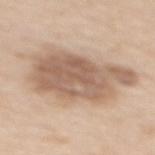Q: Automated lesion metrics?
A: an average lesion color of about L≈60 a*≈16 b*≈29 (CIELAB), about 13 CIELAB-L* units darker than the surrounding skin, and a normalized lesion–skin contrast near 8; a classifier nevus-likeness of about 10/100 and a lesion-detection confidence of about 100/100
Q: Illumination type?
A: white-light
Q: What kind of image is this?
A: total-body-photography crop, ~15 mm field of view
Q: Lesion location?
A: the mid back
Q: Who is the patient?
A: female, aged 63–67
Q: What is the lesion's diameter?
A: about 9 mm